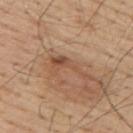Captured during whole-body skin photography for melanoma surveillance; the lesion was not biopsied. A male subject in their mid- to late 50s. The lesion is located on the upper back. A lesion tile, about 15 mm wide, cut from a 3D total-body photograph. The recorded lesion diameter is about 6 mm. Captured under white-light illumination.